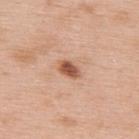Imaged during a routine full-body skin examination; the lesion was not biopsied and no histopathology is available.
This is a white-light tile.
The subject is a male aged around 60.
Automated image analysis of the tile measured a lesion area of about 4 mm², an eccentricity of roughly 0.75, and a shape-asymmetry score of about 0.15 (0 = symmetric). The software also gave a lesion color around L≈55 a*≈24 b*≈33 in CIELAB and a lesion-to-skin contrast of about 10 (normalized; higher = more distinct). The analysis additionally found a border-irregularity rating of about 1.5/10, a within-lesion color-variation index near 4/10, and peripheral color asymmetry of about 1.5.
Measured at roughly 2.5 mm in maximum diameter.
Located on the upper back.
A 15 mm close-up tile from a total-body photography series done for melanoma screening.